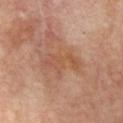Assessment: No biopsy was performed on this lesion — it was imaged during a full skin examination and was not determined to be concerning. Acquisition and patient details: A region of skin cropped from a whole-body photographic capture, roughly 15 mm wide. A male subject approximately 70 years of age. From the chest.